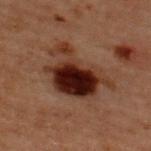Captured during whole-body skin photography for melanoma surveillance; the lesion was not biopsied. Located on the upper back. Imaged with cross-polarized lighting. A 15 mm crop from a total-body photograph taken for skin-cancer surveillance. A male patient aged around 60. Measured at roughly 7 mm in maximum diameter. The lesion-visualizer software estimated a lesion area of about 22 mm², an outline eccentricity of about 0.7 (0 = round, 1 = elongated), and a shape-asymmetry score of about 0.4 (0 = symmetric). It also reported internal color variation of about 8 on a 0–10 scale and a peripheral color-asymmetry measure near 2.5.